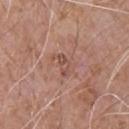<record>
  <biopsy_status>not biopsied; imaged during a skin examination</biopsy_status>
  <image>
    <source>total-body photography crop</source>
    <field_of_view_mm>15</field_of_view_mm>
  </image>
  <site>chest</site>
  <patient>
    <sex>male</sex>
    <age_approx>65</age_approx>
  </patient>
</record>